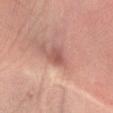Assessment: The lesion was photographed on a routine skin check and not biopsied; there is no pathology result. Image and clinical context: A male subject, about 65 years old. Located on the arm. A lesion tile, about 15 mm wide, cut from a 3D total-body photograph.